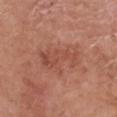Impression:
Imaged during a routine full-body skin examination; the lesion was not biopsied and no histopathology is available.
Image and clinical context:
Cropped from a whole-body photographic skin survey; the tile spans about 15 mm. An algorithmic analysis of the crop reported a lesion area of about 12 mm², a shape eccentricity near 0.9, and two-axis asymmetry of about 0.4. The software also gave a within-lesion color-variation index near 3.5/10 and peripheral color asymmetry of about 1. On the head or neck. The tile uses white-light illumination. A male subject about 60 years old.